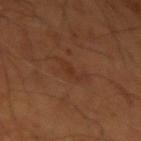Impression:
The lesion was tiled from a total-body skin photograph and was not biopsied.
Context:
About 3.5 mm across. The total-body-photography lesion software estimated a lesion–skin lightness drop of about 5 and a normalized border contrast of about 5. The tile uses cross-polarized illumination. A male subject, about 55 years old. A roughly 15 mm field-of-view crop from a total-body skin photograph. Located on the left upper arm.